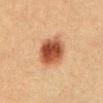| field | value |
|---|---|
| biopsy status | catalogued during a skin exam; not biopsied |
| subject | male, aged around 40 |
| location | the abdomen |
| image | total-body-photography crop, ~15 mm field of view |